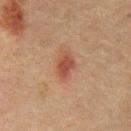A 15 mm close-up extracted from a 3D total-body photography capture.
A male patient, roughly 60 years of age.
The tile uses cross-polarized illumination.
On the chest.
The recorded lesion diameter is about 3.5 mm.